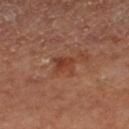{"biopsy_status": "not biopsied; imaged during a skin examination", "lesion_size": {"long_diameter_mm_approx": 2.5}, "lighting": "cross-polarized", "image": {"source": "total-body photography crop", "field_of_view_mm": 15}, "site": "right lower leg", "patient": {"sex": "female"}}